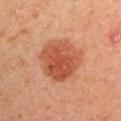The lesion was photographed on a routine skin check and not biopsied; there is no pathology result.
The lesion is on the right upper arm.
A roughly 15 mm field-of-view crop from a total-body skin photograph.
The patient is a male in their mid-40s.